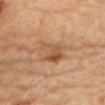biopsy status = total-body-photography surveillance lesion; no biopsy | location = the chest | subject = female, aged around 60 | illumination = cross-polarized | image = ~15 mm crop, total-body skin-cancer survey.